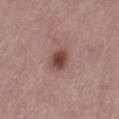{"biopsy_status": "not biopsied; imaged during a skin examination", "image": {"source": "total-body photography crop", "field_of_view_mm": 15}, "lighting": "white-light", "patient": {"sex": "male", "age_approx": 55}, "lesion_size": {"long_diameter_mm_approx": 2.5}, "site": "right thigh"}